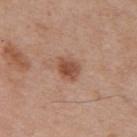Assessment:
No biopsy was performed on this lesion — it was imaged during a full skin examination and was not determined to be concerning.
Image and clinical context:
A 15 mm close-up extracted from a 3D total-body photography capture. A male subject, aged around 55. The lesion is located on the back. The recorded lesion diameter is about 3 mm. The tile uses white-light illumination.